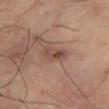<lesion>
<biopsy_status>not biopsied; imaged during a skin examination</biopsy_status>
<lesion_size>
  <long_diameter_mm_approx>3.0</long_diameter_mm_approx>
</lesion_size>
<site>left lower leg</site>
<patient>
  <sex>male</sex>
  <age_approx>45</age_approx>
</patient>
<lighting>cross-polarized</lighting>
<image>
  <source>total-body photography crop</source>
  <field_of_view_mm>15</field_of_view_mm>
</image>
</lesion>